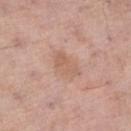<lesion>
  <biopsy_status>not biopsied; imaged during a skin examination</biopsy_status>
  <patient>
    <sex>female</sex>
    <age_approx>60</age_approx>
  </patient>
  <automated_metrics>
    <area_mm2_approx>6.5</area_mm2_approx>
    <eccentricity>0.8</eccentricity>
    <shape_asymmetry>0.25</shape_asymmetry>
    <vs_skin_darker_L>7.0</vs_skin_darker_L>
    <vs_skin_contrast_norm>5.0</vs_skin_contrast_norm>
    <color_variation_0_10>2.5</color_variation_0_10>
    <peripheral_color_asymmetry>1.0</peripheral_color_asymmetry>
  </automated_metrics>
  <image>
    <source>total-body photography crop</source>
    <field_of_view_mm>15</field_of_view_mm>
  </image>
  <lighting>white-light</lighting>
  <lesion_size>
    <long_diameter_mm_approx>4.0</long_diameter_mm_approx>
  </lesion_size>
  <site>left thigh</site>
</lesion>